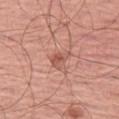No biopsy was performed on this lesion — it was imaged during a full skin examination and was not determined to be concerning. Longest diameter approximately 2.5 mm. The lesion is located on the left thigh. A male patient, roughly 65 years of age. Captured under white-light illumination. Automated image analysis of the tile measured a lesion color around L≈55 a*≈26 b*≈28 in CIELAB, a lesion–skin lightness drop of about 9, and a lesion-to-skin contrast of about 6.5 (normalized; higher = more distinct). It also reported a within-lesion color-variation index near 3/10 and peripheral color asymmetry of about 1. Cropped from a whole-body photographic skin survey; the tile spans about 15 mm.